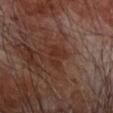This lesion was catalogued during total-body skin photography and was not selected for biopsy.